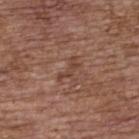biopsy status: total-body-photography surveillance lesion; no biopsy
subject: female, aged 63 to 67
acquisition: total-body-photography crop, ~15 mm field of view
anatomic site: the upper back
automated metrics: a lesion color around L≈42 a*≈20 b*≈27 in CIELAB, a lesion–skin lightness drop of about 7, and a lesion-to-skin contrast of about 6 (normalized; higher = more distinct); a nevus-likeness score of about 0/100 and a detector confidence of about 95 out of 100 that the crop contains a lesion
size: about 3 mm